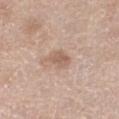Q: Is there a histopathology result?
A: catalogued during a skin exam; not biopsied
Q: How large is the lesion?
A: about 3 mm
Q: What is the anatomic site?
A: the right thigh
Q: What is the imaging modality?
A: 15 mm crop, total-body photography
Q: Patient demographics?
A: female, roughly 60 years of age
Q: Illumination type?
A: white-light illumination